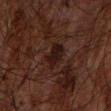follow-up: imaged on a skin check; not biopsied | diameter: ~3.5 mm (longest diameter) | patient: male, aged 63 to 67 | imaging modality: total-body-photography crop, ~15 mm field of view | TBP lesion metrics: an eccentricity of roughly 0.75 and a symmetry-axis asymmetry near 0.2; a lesion–skin lightness drop of about 6; a classifier nevus-likeness of about 0/100 and a lesion-detection confidence of about 100/100 | tile lighting: cross-polarized | site: the left forearm.